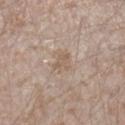Q: Was a biopsy performed?
A: total-body-photography surveillance lesion; no biopsy
Q: Who is the patient?
A: male, in their mid- to late 40s
Q: What is the lesion's diameter?
A: about 3 mm
Q: What kind of image is this?
A: total-body-photography crop, ~15 mm field of view
Q: Automated lesion metrics?
A: a footprint of about 4 mm², a shape eccentricity near 0.8, and a shape-asymmetry score of about 0.4 (0 = symmetric); a lesion color around L≈58 a*≈14 b*≈27 in CIELAB; a border-irregularity rating of about 3.5/10, a within-lesion color-variation index near 1.5/10, and a peripheral color-asymmetry measure near 0.5
Q: Where on the body is the lesion?
A: the right lower leg
Q: Illumination type?
A: white-light illumination